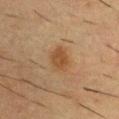This lesion was catalogued during total-body skin photography and was not selected for biopsy.
A male subject aged 53 to 57.
The lesion is located on the upper back.
A 15 mm close-up extracted from a 3D total-body photography capture.